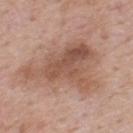Assessment:
Captured during whole-body skin photography for melanoma surveillance; the lesion was not biopsied.
Clinical summary:
Cropped from a whole-body photographic skin survey; the tile spans about 15 mm. Automated image analysis of the tile measured a border-irregularity index near 7/10 and a color-variation rating of about 5/10. The software also gave a classifier nevus-likeness of about 0/100 and lesion-presence confidence of about 100/100. A male subject roughly 55 years of age. On the back.